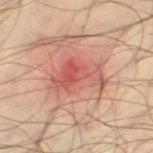Captured during whole-body skin photography for melanoma surveillance; the lesion was not biopsied. Imaged with cross-polarized lighting. A male subject, roughly 35 years of age. From the leg. The lesion-visualizer software estimated a footprint of about 14 mm² and a shape-asymmetry score of about 0.45 (0 = symmetric). It also reported a lesion color around L≈52 a*≈27 b*≈26 in CIELAB, a lesion–skin lightness drop of about 9, and a normalized lesion–skin contrast near 6.5. And it measured border irregularity of about 6 on a 0–10 scale, internal color variation of about 6.5 on a 0–10 scale, and radial color variation of about 2.5. The software also gave a classifier nevus-likeness of about 0/100 and a detector confidence of about 100 out of 100 that the crop contains a lesion. A 15 mm crop from a total-body photograph taken for skin-cancer surveillance.